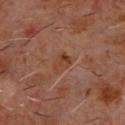<record>
  <biopsy_status>not biopsied; imaged during a skin examination</biopsy_status>
  <image>
    <source>total-body photography crop</source>
    <field_of_view_mm>15</field_of_view_mm>
  </image>
  <site>chest</site>
  <patient>
    <sex>male</sex>
    <age_approx>60</age_approx>
  </patient>
</record>